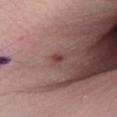Impression:
This lesion was catalogued during total-body skin photography and was not selected for biopsy.
Acquisition and patient details:
A lesion tile, about 15 mm wide, cut from a 3D total-body photograph. The lesion is on the right lower leg. Longest diameter approximately 2 mm. An algorithmic analysis of the crop reported a lesion area of about 1.5 mm² and a shape-asymmetry score of about 0.2 (0 = symmetric). The software also gave an average lesion color of about L≈41 a*≈21 b*≈21 (CIELAB), about 10 CIELAB-L* units darker than the surrounding skin, and a normalized lesion–skin contrast near 8. And it measured border irregularity of about 2 on a 0–10 scale, a color-variation rating of about 0/10, and a peripheral color-asymmetry measure near 0. The software also gave a classifier nevus-likeness of about 30/100 and a lesion-detection confidence of about 100/100. The patient is a female about 45 years old. This is a white-light tile.